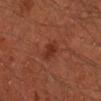Impression:
Part of a total-body skin-imaging series; this lesion was reviewed on a skin check and was not flagged for biopsy.
Background:
A 15 mm close-up extracted from a 3D total-body photography capture. A male patient approximately 45 years of age. The lesion is located on the right forearm.